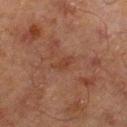Imaged during a routine full-body skin examination; the lesion was not biopsied and no histopathology is available. A male subject, about 65 years old. A 15 mm close-up tile from a total-body photography series done for melanoma screening. Longest diameter approximately 2.5 mm. This is a cross-polarized tile. The lesion is located on the left lower leg.